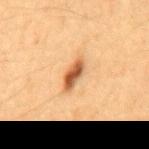{
  "biopsy_status": "not biopsied; imaged during a skin examination",
  "patient": {
    "sex": "male",
    "age_approx": 65
  },
  "automated_metrics": {
    "area_mm2_approx": 5.5,
    "eccentricity": 0.9,
    "shape_asymmetry": 0.3,
    "border_irregularity_0_10": 3.0,
    "color_variation_0_10": 4.0,
    "peripheral_color_asymmetry": 1.0,
    "nevus_likeness_0_100": 95
  },
  "image": {
    "source": "total-body photography crop",
    "field_of_view_mm": 15
  },
  "site": "mid back"
}